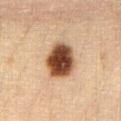Recorded during total-body skin imaging; not selected for excision or biopsy. Located on the abdomen. A region of skin cropped from a whole-body photographic capture, roughly 15 mm wide. The subject is a female approximately 30 years of age. Imaged with cross-polarized lighting. The lesion-visualizer software estimated a lesion area of about 14 mm². The software also gave border irregularity of about 1.5 on a 0–10 scale, a within-lesion color-variation index near 6.5/10, and radial color variation of about 1.5. About 4.5 mm across.